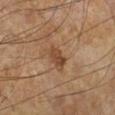{
  "site": "left lower leg",
  "image": {
    "source": "total-body photography crop",
    "field_of_view_mm": 15
  },
  "patient": {
    "sex": "male",
    "age_approx": 65
  }
}